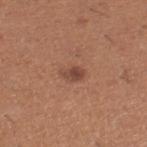<case>
<biopsy_status>not biopsied; imaged during a skin examination</biopsy_status>
<site>leg</site>
<patient>
  <sex>male</sex>
  <age_approx>40</age_approx>
</patient>
<image>
  <source>total-body photography crop</source>
  <field_of_view_mm>15</field_of_view_mm>
</image>
<lighting>white-light</lighting>
</case>